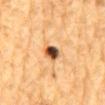Imaged during a routine full-body skin examination; the lesion was not biopsied and no histopathology is available. On the mid back. A 15 mm close-up tile from a total-body photography series done for melanoma screening. Imaged with cross-polarized lighting. The subject is a male in their mid- to late 80s or older. The total-body-photography lesion software estimated an area of roughly 4.5 mm², an eccentricity of roughly 0.75, and a shape-asymmetry score of about 0.2 (0 = symmetric). The software also gave a lesion–skin lightness drop of about 21 and a normalized border contrast of about 15. The software also gave a nevus-likeness score of about 100/100.